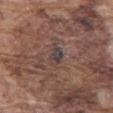A roughly 15 mm field-of-view crop from a total-body skin photograph.
The lesion's longest dimension is about 3 mm.
The subject is a male about 75 years old.
From the front of the torso.